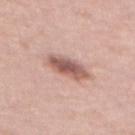workup: catalogued during a skin exam; not biopsied | site: the upper back | subject: female, aged 63–67 | imaging modality: 15 mm crop, total-body photography | automated lesion analysis: a mean CIELAB color near L≈57 a*≈21 b*≈24, a lesion–skin lightness drop of about 14, and a normalized border contrast of about 9; a classifier nevus-likeness of about 65/100 and lesion-presence confidence of about 100/100.